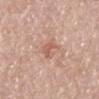<record>
  <biopsy_status>not biopsied; imaged during a skin examination</biopsy_status>
  <patient>
    <sex>male</sex>
    <age_approx>80</age_approx>
  </patient>
  <site>mid back</site>
  <image>
    <source>total-body photography crop</source>
    <field_of_view_mm>15</field_of_view_mm>
  </image>
  <lighting>white-light</lighting>
</record>